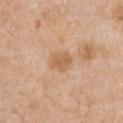Recorded during total-body skin imaging; not selected for excision or biopsy. The lesion is located on the chest. A 15 mm close-up tile from a total-body photography series done for melanoma screening. A female subject, approximately 70 years of age.